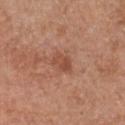* notes — catalogued during a skin exam; not biopsied
* subject — female, aged approximately 50
* lesion size — ≈3 mm
* imaging modality — 15 mm crop, total-body photography
* body site — the chest